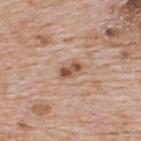* biopsy status · total-body-photography surveillance lesion; no biopsy
* subject · male, aged 63–67
* acquisition · total-body-photography crop, ~15 mm field of view
* size · ~3 mm (longest diameter)
* location · the upper back